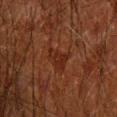This lesion was catalogued during total-body skin photography and was not selected for biopsy.
A 15 mm crop from a total-body photograph taken for skin-cancer surveillance.
Imaged with cross-polarized lighting.
The total-body-photography lesion software estimated a footprint of about 4.5 mm² and a shape-asymmetry score of about 0.45 (0 = symmetric).
The subject is a male aged approximately 60.
The lesion is on the right forearm.